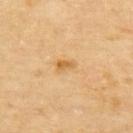Findings:
- tile lighting · cross-polarized illumination
- lesion size · about 4.5 mm
- imaging modality · total-body-photography crop, ~15 mm field of view
- anatomic site · the upper back
- subject · female, aged 68–72